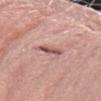{
  "biopsy_status": "not biopsied; imaged during a skin examination",
  "image": {
    "source": "total-body photography crop",
    "field_of_view_mm": 15
  },
  "patient": {
    "sex": "female",
    "age_approx": 60
  },
  "site": "left forearm"
}